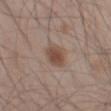Case summary:
– notes · no biopsy performed (imaged during a skin exam)
– TBP lesion metrics · a lesion area of about 9.5 mm², a shape eccentricity near 0.7, and two-axis asymmetry of about 0.15; border irregularity of about 1.5 on a 0–10 scale
– anatomic site · the left thigh
– lighting · white-light illumination
– acquisition · ~15 mm crop, total-body skin-cancer survey
– subject · male, aged around 45
– size · ≈4.5 mm